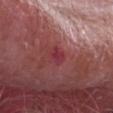Impression: No biopsy was performed on this lesion — it was imaged during a full skin examination and was not determined to be concerning. Clinical summary: A 15 mm close-up tile from a total-body photography series done for melanoma screening. The lesion is on the head or neck. The subject is a male aged approximately 65.